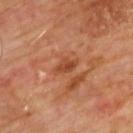A 15 mm close-up tile from a total-body photography series done for melanoma screening.
On the upper back.
Automated tile analysis of the lesion measured an average lesion color of about L≈45 a*≈27 b*≈35 (CIELAB) and a lesion–skin lightness drop of about 9. The analysis additionally found a border-irregularity rating of about 3/10 and peripheral color asymmetry of about 1.
Measured at roughly 3 mm in maximum diameter.
Imaged with cross-polarized lighting.
A male subject, aged around 60.